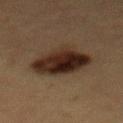Imaged during a routine full-body skin examination; the lesion was not biopsied and no histopathology is available. A 15 mm close-up extracted from a 3D total-body photography capture. A female subject, aged approximately 40. The total-body-photography lesion software estimated about 12 CIELAB-L* units darker than the surrounding skin and a normalized lesion–skin contrast near 14. The analysis additionally found a nevus-likeness score of about 95/100. Longest diameter approximately 5.5 mm. The lesion is located on the mid back.